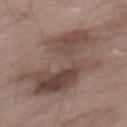workup: total-body-photography surveillance lesion; no biopsy
image source: ~15 mm tile from a whole-body skin photo
subject: male, aged approximately 55
anatomic site: the back